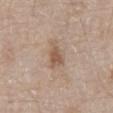| feature | finding |
|---|---|
| follow-up | no biopsy performed (imaged during a skin exam) |
| subject | male, roughly 80 years of age |
| location | the abdomen |
| imaging modality | ~15 mm tile from a whole-body skin photo |